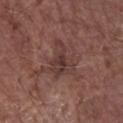Recorded during total-body skin imaging; not selected for excision or biopsy. About 4.5 mm across. From the arm. The subject is a male aged 68 to 72. A roughly 15 mm field-of-view crop from a total-body skin photograph.